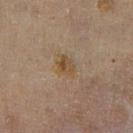| key | value |
|---|---|
| biopsy status | catalogued during a skin exam; not biopsied |
| size | ~3 mm (longest diameter) |
| subject | female, approximately 60 years of age |
| automated metrics | an average lesion color of about L≈48 a*≈14 b*≈32 (CIELAB), about 6 CIELAB-L* units darker than the surrounding skin, and a normalized lesion–skin contrast near 7; internal color variation of about 5.5 on a 0–10 scale and a peripheral color-asymmetry measure near 2; an automated nevus-likeness rating near 25 out of 100 and a lesion-detection confidence of about 100/100 |
| body site | the right lower leg |
| image source | 15 mm crop, total-body photography |
| illumination | cross-polarized illumination |